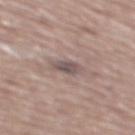Part of a total-body skin-imaging series; this lesion was reviewed on a skin check and was not flagged for biopsy.
The lesion is located on the mid back.
Longest diameter approximately 2.5 mm.
Imaged with white-light lighting.
The patient is a male in their mid-70s.
A 15 mm close-up tile from a total-body photography series done for melanoma screening.